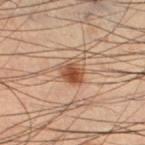• follow-up: total-body-photography surveillance lesion; no biopsy
• image: ~15 mm crop, total-body skin-cancer survey
• diameter: about 3 mm
• location: the left lower leg
• subject: male, aged around 55
• lighting: cross-polarized